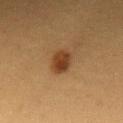Q: Was a biopsy performed?
A: total-body-photography surveillance lesion; no biopsy
Q: Patient demographics?
A: female, aged 38–42
Q: What kind of image is this?
A: total-body-photography crop, ~15 mm field of view
Q: Automated lesion metrics?
A: a classifier nevus-likeness of about 100/100 and a detector confidence of about 100 out of 100 that the crop contains a lesion
Q: Lesion location?
A: the chest
Q: What lighting was used for the tile?
A: cross-polarized